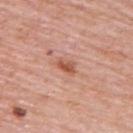Notes:
• workup: total-body-photography surveillance lesion; no biopsy
• acquisition: 15 mm crop, total-body photography
• lighting: white-light illumination
• subject: male, aged approximately 80
• lesion size: ~3 mm (longest diameter)
• location: the upper back